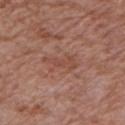Assessment:
No biopsy was performed on this lesion — it was imaged during a full skin examination and was not determined to be concerning.
Acquisition and patient details:
The lesion is located on the left upper arm. A 15 mm crop from a total-body photograph taken for skin-cancer surveillance. A male subject, aged 68–72. The total-body-photography lesion software estimated a border-irregularity rating of about 6.5/10, a color-variation rating of about 1/10, and peripheral color asymmetry of about 0.5. The lesion's longest dimension is about 4.5 mm.